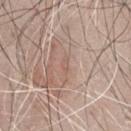Context: An algorithmic analysis of the crop reported a lesion area of about 1.5 mm² and a shape-asymmetry score of about 0.5 (0 = symmetric). And it measured a lesion color around L≈58 a*≈18 b*≈26 in CIELAB, about 5 CIELAB-L* units darker than the surrounding skin, and a lesion-to-skin contrast of about 3.5 (normalized; higher = more distinct). It also reported a border-irregularity rating of about 4/10, a color-variation rating of about 0/10, and peripheral color asymmetry of about 0. It also reported an automated nevus-likeness rating near 0 out of 100. The lesion is located on the front of the torso. The subject is a male in their mid-60s. About 1.5 mm across. A 15 mm close-up tile from a total-body photography series done for melanoma screening. Imaged with white-light lighting.